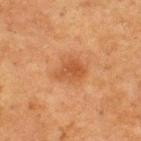lighting: cross-polarized
patient:
  sex: male
  age_approx: 75
site: back
lesion_size:
  long_diameter_mm_approx: 4.0
image:
  source: total-body photography crop
  field_of_view_mm: 15
automated_metrics:
  border_irregularity_0_10: 3.0
  color_variation_0_10: 3.0
  peripheral_color_asymmetry: 1.0